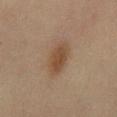Findings:
- follow-up — no biopsy performed (imaged during a skin exam)
- imaging modality — total-body-photography crop, ~15 mm field of view
- image-analysis metrics — an area of roughly 7 mm², an eccentricity of roughly 0.85, and a symmetry-axis asymmetry near 0.2; a lesion color around L≈40 a*≈15 b*≈28 in CIELAB and a lesion–skin lightness drop of about 8; an automated nevus-likeness rating near 100 out of 100 and a detector confidence of about 100 out of 100 that the crop contains a lesion
- body site — the mid back
- patient — female, roughly 60 years of age